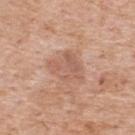No biopsy was performed on this lesion — it was imaged during a full skin examination and was not determined to be concerning. The lesion's longest dimension is about 4.5 mm. The lesion is located on the back. A 15 mm crop from a total-body photograph taken for skin-cancer surveillance. Imaged with white-light lighting. A female patient, in their 40s.